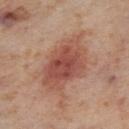follow-up: total-body-photography surveillance lesion; no biopsy | image-analysis metrics: a footprint of about 23 mm², an outline eccentricity of about 0.85 (0 = round, 1 = elongated), and a symmetry-axis asymmetry near 0.2; a lesion–skin lightness drop of about 11 and a normalized border contrast of about 8; an automated nevus-likeness rating near 85 out of 100 | location: the right thigh | acquisition: ~15 mm crop, total-body skin-cancer survey | lesion size: ≈8 mm | patient: female, about 55 years old.